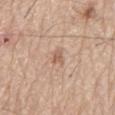Assessment:
This lesion was catalogued during total-body skin photography and was not selected for biopsy.
Clinical summary:
The lesion is located on the mid back. The tile uses white-light illumination. The subject is a male aged 78 to 82. Automated tile analysis of the lesion measured an average lesion color of about L≈60 a*≈19 b*≈30 (CIELAB) and a lesion-to-skin contrast of about 6 (normalized; higher = more distinct). The analysis additionally found an automated nevus-likeness rating near 0 out of 100 and a lesion-detection confidence of about 100/100. A close-up tile cropped from a whole-body skin photograph, about 15 mm across. About 2.5 mm across.